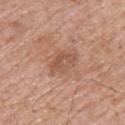This lesion was catalogued during total-body skin photography and was not selected for biopsy. A male patient, aged 68 to 72. A lesion tile, about 15 mm wide, cut from a 3D total-body photograph. The tile uses white-light illumination. Longest diameter approximately 4 mm. Automated tile analysis of the lesion measured border irregularity of about 3.5 on a 0–10 scale, internal color variation of about 3.5 on a 0–10 scale, and peripheral color asymmetry of about 1. The lesion is located on the upper back.